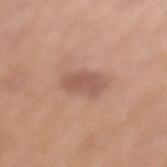Findings:
– notes · imaged on a skin check; not biopsied
– location · the left lower leg
– image source · 15 mm crop, total-body photography
– patient · male, aged approximately 40